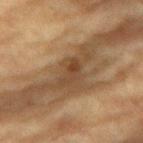lesion_size:
  long_diameter_mm_approx: 3.5
site: back
image:
  source: total-body photography crop
  field_of_view_mm: 15
patient:
  sex: male
  age_approx: 85+